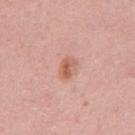| key | value |
|---|---|
| workup | no biopsy performed (imaged during a skin exam) |
| patient | male, aged around 55 |
| automated lesion analysis | a mean CIELAB color near L≈60 a*≈25 b*≈30, a lesion–skin lightness drop of about 10, and a lesion-to-skin contrast of about 7.5 (normalized; higher = more distinct); border irregularity of about 2.5 on a 0–10 scale, a within-lesion color-variation index near 4/10, and peripheral color asymmetry of about 1.5; a classifier nevus-likeness of about 90/100 and lesion-presence confidence of about 100/100 |
| lesion size | ≈2.5 mm |
| image | total-body-photography crop, ~15 mm field of view |
| anatomic site | the abdomen |